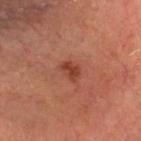Case summary:
– notes — imaged on a skin check; not biopsied
– site — the head or neck
– imaging modality — total-body-photography crop, ~15 mm field of view
– subject — male, aged approximately 65
– automated metrics — border irregularity of about 3 on a 0–10 scale, a color-variation rating of about 1.5/10, and peripheral color asymmetry of about 0.5
– diameter — ≈2.5 mm
– lighting — cross-polarized